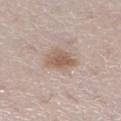No biopsy was performed on this lesion — it was imaged during a full skin examination and was not determined to be concerning.
From the right lower leg.
The subject is a female in their 40s.
Cropped from a whole-body photographic skin survey; the tile spans about 15 mm.
Approximately 4 mm at its widest.
Automated tile analysis of the lesion measured a border-irregularity index near 2/10. It also reported an automated nevus-likeness rating near 85 out of 100.
The tile uses white-light illumination.